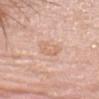This lesion was catalogued during total-body skin photography and was not selected for biopsy. This is a white-light tile. This image is a 15 mm lesion crop taken from a total-body photograph. Approximately 3.5 mm at its widest. The subject is a female roughly 60 years of age. The lesion-visualizer software estimated an area of roughly 5.5 mm², an outline eccentricity of about 0.8 (0 = round, 1 = elongated), and a shape-asymmetry score of about 0.35 (0 = symmetric). And it measured about 7 CIELAB-L* units darker than the surrounding skin and a lesion-to-skin contrast of about 5 (normalized; higher = more distinct). It also reported a nevus-likeness score of about 0/100 and a lesion-detection confidence of about 100/100. On the head or neck.